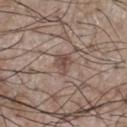Case summary:
* follow-up — total-body-photography surveillance lesion; no biopsy
* subject — male, approximately 75 years of age
* TBP lesion metrics — a footprint of about 4 mm², a shape eccentricity near 0.5, and two-axis asymmetry of about 0.25; roughly 10 lightness units darker than nearby skin; lesion-presence confidence of about 100/100
* body site — the chest
* image source — ~15 mm crop, total-body skin-cancer survey